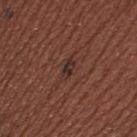workup: total-body-photography surveillance lesion; no biopsy | image source: ~15 mm tile from a whole-body skin photo | patient: male, aged 43 to 47 | body site: the upper back | automated lesion analysis: an area of roughly 3 mm², an outline eccentricity of about 0.8 (0 = round, 1 = elongated), and two-axis asymmetry of about 0.3; a mean CIELAB color near L≈30 a*≈18 b*≈20 and a lesion-to-skin contrast of about 8.5 (normalized; higher = more distinct) | diameter: about 2.5 mm | lighting: white-light.